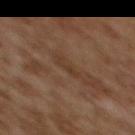<lesion>
<biopsy_status>not biopsied; imaged during a skin examination</biopsy_status>
<patient>
  <sex>female</sex>
  <age_approx>60</age_approx>
</patient>
<image>
  <source>total-body photography crop</source>
  <field_of_view_mm>15</field_of_view_mm>
</image>
<site>upper back</site>
</lesion>